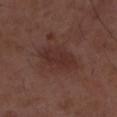Part of a total-body skin-imaging series; this lesion was reviewed on a skin check and was not flagged for biopsy.
The lesion's longest dimension is about 4.5 mm.
A male patient, about 50 years old.
Automated tile analysis of the lesion measured a border-irregularity index near 2.5/10 and a peripheral color-asymmetry measure near 0.5. It also reported a nevus-likeness score of about 5/100 and lesion-presence confidence of about 100/100.
A close-up tile cropped from a whole-body skin photograph, about 15 mm across.
Imaged with white-light lighting.